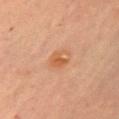Assessment:
The lesion was tiled from a total-body skin photograph and was not biopsied.
Context:
Imaged with cross-polarized lighting. Measured at roughly 2.5 mm in maximum diameter. The subject is a female aged approximately 60. The lesion is on the left upper arm. Automated image analysis of the tile measured a lesion–skin lightness drop of about 7 and a normalized border contrast of about 6.5. The software also gave a classifier nevus-likeness of about 60/100. A region of skin cropped from a whole-body photographic capture, roughly 15 mm wide.